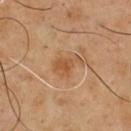{
  "lesion_size": {
    "long_diameter_mm_approx": 3.5
  },
  "patient": {
    "sex": "male",
    "age_approx": 50
  },
  "automated_metrics": {
    "area_mm2_approx": 5.0,
    "eccentricity": 0.75,
    "shape_asymmetry": 0.4,
    "cielab_L": 52,
    "cielab_a": 21,
    "cielab_b": 37,
    "vs_skin_darker_L": 8.0,
    "vs_skin_contrast_norm": 6.5,
    "border_irregularity_0_10": 4.5,
    "color_variation_0_10": 2.0,
    "peripheral_color_asymmetry": 0.5,
    "nevus_likeness_0_100": 55,
    "lesion_detection_confidence_0_100": 100
  },
  "site": "chest",
  "lighting": "cross-polarized",
  "image": {
    "source": "total-body photography crop",
    "field_of_view_mm": 15
  }
}